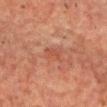This image is a 15 mm lesion crop taken from a total-body photograph.
This is a cross-polarized tile.
Automated image analysis of the tile measured a lesion area of about 3.5 mm², an eccentricity of roughly 0.85, and two-axis asymmetry of about 0.25. And it measured a classifier nevus-likeness of about 0/100 and a lesion-detection confidence of about 95/100.
On the chest.
About 2.5 mm across.
A male patient, aged 58–62.